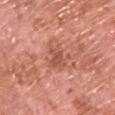Recorded during total-body skin imaging; not selected for excision or biopsy.